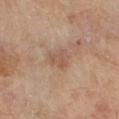workup — imaged on a skin check; not biopsied.